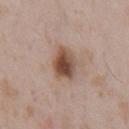biopsy status: no biopsy performed (imaged during a skin exam) | patient: male, in their 50s | image source: total-body-photography crop, ~15 mm field of view | automated metrics: a lesion area of about 8.5 mm², an outline eccentricity of about 0.6 (0 = round, 1 = elongated), and a symmetry-axis asymmetry near 0.2; a border-irregularity rating of about 2/10, a within-lesion color-variation index near 6.5/10, and peripheral color asymmetry of about 2; an automated nevus-likeness rating near 95 out of 100 | body site: the chest.